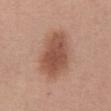Located on the front of the torso.
The subject is a female aged 58–62.
A lesion tile, about 15 mm wide, cut from a 3D total-body photograph.